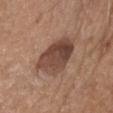biopsy_status: not biopsied; imaged during a skin examination
image:
  source: total-body photography crop
  field_of_view_mm: 15
lighting: white-light
patient:
  sex: male
  age_approx: 55
lesion_size:
  long_diameter_mm_approx: 5.0
site: head or neck